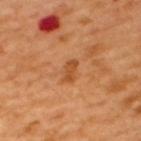No biopsy was performed on this lesion — it was imaged during a full skin examination and was not determined to be concerning. About 2.5 mm across. This is a cross-polarized tile. A male patient about 50 years old. Located on the upper back. The total-body-photography lesion software estimated an outline eccentricity of about 0.9 (0 = round, 1 = elongated) and a shape-asymmetry score of about 0.25 (0 = symmetric). The analysis additionally found border irregularity of about 3 on a 0–10 scale and a peripheral color-asymmetry measure near 0. A 15 mm close-up extracted from a 3D total-body photography capture.